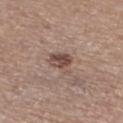notes: total-body-photography surveillance lesion; no biopsy
TBP lesion metrics: a footprint of about 6 mm², an outline eccentricity of about 0.75 (0 = round, 1 = elongated), and a shape-asymmetry score of about 0.4 (0 = symmetric); a border-irregularity index near 4/10, a color-variation rating of about 4.5/10, and a peripheral color-asymmetry measure near 2; a nevus-likeness score of about 75/100
site: the left lower leg
lighting: white-light
lesion size: ≈3.5 mm
acquisition: 15 mm crop, total-body photography
patient: female, aged 73 to 77